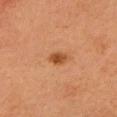No biopsy was performed on this lesion — it was imaged during a full skin examination and was not determined to be concerning.